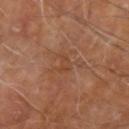  biopsy_status: not biopsied; imaged during a skin examination
  patient:
    sex: male
    age_approx: 70
  lighting: cross-polarized
  lesion_size:
    long_diameter_mm_approx: 2.5
  site: right forearm
  image:
    source: total-body photography crop
    field_of_view_mm: 15